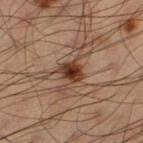On the leg. A region of skin cropped from a whole-body photographic capture, roughly 15 mm wide. A male subject aged 58 to 62.